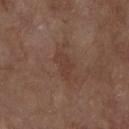anatomic site: the chest
lesion diameter: ~3.5 mm (longest diameter)
image-analysis metrics: about 5 CIELAB-L* units darker than the surrounding skin; a border-irregularity rating of about 4/10 and a color-variation rating of about 1/10
subject: male, approximately 80 years of age
acquisition: ~15 mm tile from a whole-body skin photo